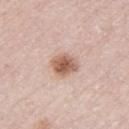Longest diameter approximately 3 mm. A 15 mm close-up extracted from a 3D total-body photography capture. The total-body-photography lesion software estimated a footprint of about 7 mm² and a symmetry-axis asymmetry near 0.15. And it measured a border-irregularity index near 1.5/10 and internal color variation of about 4 on a 0–10 scale. And it measured a lesion-detection confidence of about 100/100. From the left lower leg. The subject is a male in their 80s.